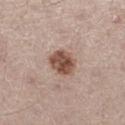Part of a total-body skin-imaging series; this lesion was reviewed on a skin check and was not flagged for biopsy. This is a white-light tile. On the left thigh. Measured at roughly 3.5 mm in maximum diameter. Automated image analysis of the tile measured about 15 CIELAB-L* units darker than the surrounding skin and a normalized lesion–skin contrast near 10.5. The analysis additionally found a color-variation rating of about 5/10. And it measured an automated nevus-likeness rating near 90 out of 100 and a lesion-detection confidence of about 100/100. A male subject, about 65 years old. A 15 mm close-up tile from a total-body photography series done for melanoma screening.